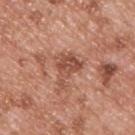follow-up: total-body-photography surveillance lesion; no biopsy
illumination: white-light
site: the upper back
automated metrics: an outline eccentricity of about 0.6 (0 = round, 1 = elongated) and two-axis asymmetry of about 0.55; border irregularity of about 6.5 on a 0–10 scale, internal color variation of about 3.5 on a 0–10 scale, and radial color variation of about 1.5
subject: male, in their mid- to late 50s
image source: ~15 mm crop, total-body skin-cancer survey
lesion diameter: about 4 mm